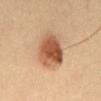Findings:
- follow-up: total-body-photography surveillance lesion; no biopsy
- subject: male, aged 58–62
- body site: the front of the torso
- imaging modality: ~15 mm tile from a whole-body skin photo